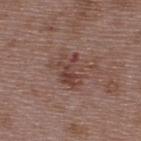biopsy status = catalogued during a skin exam; not biopsied | body site = the back | lighting = white-light | patient = male, in their 50s | diameter = about 4.5 mm | image source = total-body-photography crop, ~15 mm field of view.